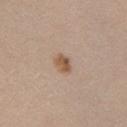Impression: No biopsy was performed on this lesion — it was imaged during a full skin examination and was not determined to be concerning. Image and clinical context: The lesion is on the left upper arm. A female subject in their 30s. A close-up tile cropped from a whole-body skin photograph, about 15 mm across.